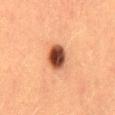biopsy status: imaged on a skin check; not biopsied
tile lighting: cross-polarized illumination
automated lesion analysis: a lesion color around L≈41 a*≈23 b*≈29 in CIELAB, a lesion–skin lightness drop of about 18, and a normalized border contrast of about 13.5; a nevus-likeness score of about 100/100 and a detector confidence of about 100 out of 100 that the crop contains a lesion
size: ~3.5 mm (longest diameter)
subject: female, in their mid- to late 50s
anatomic site: the mid back
imaging modality: total-body-photography crop, ~15 mm field of view